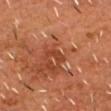Findings:
* follow-up · catalogued during a skin exam; not biopsied
* patient · male, aged 53 to 57
* site · the head or neck
* imaging modality · ~15 mm crop, total-body skin-cancer survey